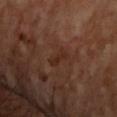| feature | finding |
|---|---|
| notes | catalogued during a skin exam; not biopsied |
| automated lesion analysis | a classifier nevus-likeness of about 0/100 and a lesion-detection confidence of about 100/100 |
| anatomic site | the upper back |
| lesion size | ~3 mm (longest diameter) |
| acquisition | ~15 mm tile from a whole-body skin photo |
| lighting | cross-polarized |
| patient | male, aged 68 to 72 |